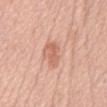No biopsy was performed on this lesion — it was imaged during a full skin examination and was not determined to be concerning. Longest diameter approximately 3 mm. A close-up tile cropped from a whole-body skin photograph, about 15 mm across. A female subject, in their mid- to late 50s. The tile uses white-light illumination. On the chest.